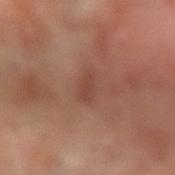Captured during whole-body skin photography for melanoma surveillance; the lesion was not biopsied. From the left forearm. Imaged with cross-polarized lighting. A 15 mm close-up extracted from a 3D total-body photography capture. An algorithmic analysis of the crop reported a lesion area of about 4 mm². The analysis additionally found a mean CIELAB color near L≈44 a*≈23 b*≈27, roughly 6 lightness units darker than nearby skin, and a normalized border contrast of about 5. And it measured a border-irregularity index near 2.5/10 and a within-lesion color-variation index near 1.5/10. A female subject aged around 75.